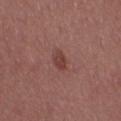Impression:
Imaged during a routine full-body skin examination; the lesion was not biopsied and no histopathology is available.
Background:
Located on the left thigh. A lesion tile, about 15 mm wide, cut from a 3D total-body photograph. The patient is a female aged 38 to 42.